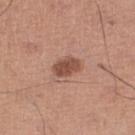Assessment: Captured during whole-body skin photography for melanoma surveillance; the lesion was not biopsied. Background: A male subject aged approximately 55. On the right lower leg. Approximately 3.5 mm at its widest. The lesion-visualizer software estimated a lesion-to-skin contrast of about 8.5 (normalized; higher = more distinct). It also reported a border-irregularity index near 2/10 and radial color variation of about 1. And it measured a classifier nevus-likeness of about 75/100 and a lesion-detection confidence of about 100/100. A close-up tile cropped from a whole-body skin photograph, about 15 mm across.